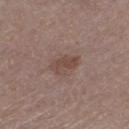This lesion was catalogued during total-body skin photography and was not selected for biopsy. The total-body-photography lesion software estimated a footprint of about 5.5 mm², a shape eccentricity near 0.75, and a shape-asymmetry score of about 0.25 (0 = symmetric). The lesion's longest dimension is about 3 mm. Captured under white-light illumination. A female subject, in their 50s. Located on the left thigh. A lesion tile, about 15 mm wide, cut from a 3D total-body photograph.